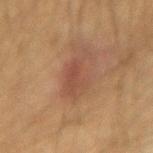| key | value |
|---|---|
| follow-up | total-body-photography surveillance lesion; no biopsy |
| illumination | cross-polarized |
| size | ~5 mm (longest diameter) |
| patient | male, about 50 years old |
| image source | ~15 mm tile from a whole-body skin photo |
| site | the right upper arm |
| TBP lesion metrics | a shape eccentricity near 0.85 and a shape-asymmetry score of about 0.4 (0 = symmetric); an automated nevus-likeness rating near 75 out of 100 and a lesion-detection confidence of about 100/100 |